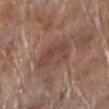Q: Is there a histopathology result?
A: imaged on a skin check; not biopsied
Q: What did automated image analysis measure?
A: a footprint of about 9 mm² and a shape-asymmetry score of about 0.45 (0 = symmetric); a border-irregularity index near 6.5/10 and a within-lesion color-variation index near 3/10; an automated nevus-likeness rating near 0 out of 100 and a detector confidence of about 95 out of 100 that the crop contains a lesion
Q: What are the patient's age and sex?
A: male, in their 70s
Q: Lesion location?
A: the left lower leg
Q: What lighting was used for the tile?
A: white-light illumination
Q: What is the imaging modality?
A: ~15 mm crop, total-body skin-cancer survey